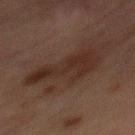  biopsy_status: not biopsied; imaged during a skin examination